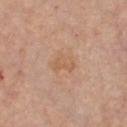<tbp_lesion>
<biopsy_status>not biopsied; imaged during a skin examination</biopsy_status>
<site>chest</site>
<patient>
  <sex>male</sex>
  <age_approx>60</age_approx>
</patient>
<automated_metrics>
  <lesion_detection_confidence_0_100>100</lesion_detection_confidence_0_100>
</automated_metrics>
<image>
  <source>total-body photography crop</source>
  <field_of_view_mm>15</field_of_view_mm>
</image>
</tbp_lesion>